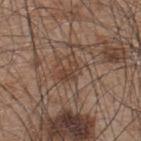Impression: Imaged during a routine full-body skin examination; the lesion was not biopsied and no histopathology is available. Acquisition and patient details: This image is a 15 mm lesion crop taken from a total-body photograph. Located on the upper back. The patient is a male in their mid-40s.